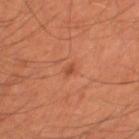<case>
<biopsy_status>not biopsied; imaged during a skin examination</biopsy_status>
<patient>
  <sex>male</sex>
  <age_approx>55</age_approx>
</patient>
<lesion_size>
  <long_diameter_mm_approx>1.5</long_diameter_mm_approx>
</lesion_size>
<lighting>cross-polarized</lighting>
<image>
  <source>total-body photography crop</source>
  <field_of_view_mm>15</field_of_view_mm>
</image>
<site>leg</site>
</case>